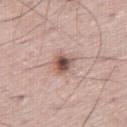Assessment:
This lesion was catalogued during total-body skin photography and was not selected for biopsy.